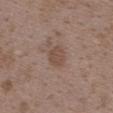This lesion was catalogued during total-body skin photography and was not selected for biopsy. A female patient, aged around 35. The recorded lesion diameter is about 3 mm. The lesion is located on the upper back. A close-up tile cropped from a whole-body skin photograph, about 15 mm across. Captured under white-light illumination. Automated image analysis of the tile measured a mean CIELAB color near L≈48 a*≈16 b*≈25, a lesion–skin lightness drop of about 7, and a normalized border contrast of about 6. And it measured a border-irregularity index near 1.5/10, internal color variation of about 1.5 on a 0–10 scale, and a peripheral color-asymmetry measure near 0.5.